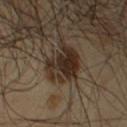Q: Is there a histopathology result?
A: catalogued during a skin exam; not biopsied
Q: What is the lesion's diameter?
A: about 4.5 mm
Q: What is the imaging modality?
A: ~15 mm crop, total-body skin-cancer survey
Q: What is the anatomic site?
A: the right upper arm
Q: Automated lesion metrics?
A: a footprint of about 13 mm²; border irregularity of about 4 on a 0–10 scale, a within-lesion color-variation index near 5.5/10, and peripheral color asymmetry of about 2
Q: Who is the patient?
A: male, aged 53 to 57